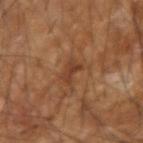<tbp_lesion>
<biopsy_status>not biopsied; imaged during a skin examination</biopsy_status>
<site>right forearm</site>
<lighting>cross-polarized</lighting>
<patient>
  <sex>male</sex>
  <age_approx>55</age_approx>
</patient>
<image>
  <source>total-body photography crop</source>
  <field_of_view_mm>15</field_of_view_mm>
</image>
<lesion_size>
  <long_diameter_mm_approx>2.5</long_diameter_mm_approx>
</lesion_size>
</tbp_lesion>